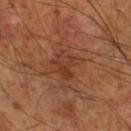Imaged during a routine full-body skin examination; the lesion was not biopsied and no histopathology is available.
Cropped from a whole-body photographic skin survey; the tile spans about 15 mm.
A male patient, roughly 60 years of age.
About 3.5 mm across.
Automated tile analysis of the lesion measured a lesion color around L≈37 a*≈23 b*≈31 in CIELAB, roughly 6 lightness units darker than nearby skin, and a lesion-to-skin contrast of about 5.5 (normalized; higher = more distinct). And it measured a border-irregularity rating of about 5/10 and peripheral color asymmetry of about 1.5.
From the right lower leg.
The tile uses cross-polarized illumination.